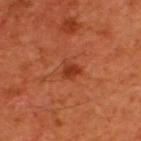{"biopsy_status": "not biopsied; imaged during a skin examination", "site": "back", "patient": {"sex": "male", "age_approx": 60}, "lighting": "cross-polarized", "image": {"source": "total-body photography crop", "field_of_view_mm": 15}, "lesion_size": {"long_diameter_mm_approx": 2.0}}